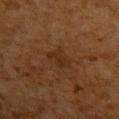  biopsy_status: not biopsied; imaged during a skin examination
  patient:
    sex: male
    age_approx: 60
  site: upper back
  lesion_size:
    long_diameter_mm_approx: 3.0
  automated_metrics:
    cielab_L: 23
    cielab_a: 16
    cielab_b: 26
    vs_skin_darker_L: 4.0
    vs_skin_contrast_norm: 5.5
    nevus_likeness_0_100: 0
    lesion_detection_confidence_0_100: 100
  image:
    source: total-body photography crop
    field_of_view_mm: 15